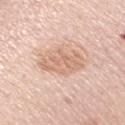biopsy_status: not biopsied; imaged during a skin examination
site: right upper arm
lighting: white-light
image:
  source: total-body photography crop
  field_of_view_mm: 15
patient:
  sex: female
  age_approx: 60